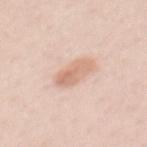follow-up: catalogued during a skin exam; not biopsied | imaging modality: 15 mm crop, total-body photography | location: the mid back | subject: female, aged 43–47 | lighting: white-light illumination.